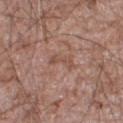Imaged during a routine full-body skin examination; the lesion was not biopsied and no histopathology is available. Longest diameter approximately 3.5 mm. A 15 mm close-up tile from a total-body photography series done for melanoma screening. A male subject aged 58 to 62. The lesion is located on the right lower leg. The tile uses white-light illumination.